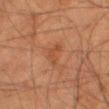• follow-up: no biopsy performed (imaged during a skin exam)
• acquisition: total-body-photography crop, ~15 mm field of view
• location: the right thigh
• subject: male, about 80 years old